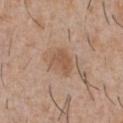* workup · imaged on a skin check; not biopsied